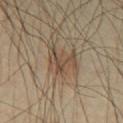Automated tile analysis of the lesion measured a lesion area of about 8.5 mm², an outline eccentricity of about 0.55 (0 = round, 1 = elongated), and a symmetry-axis asymmetry near 0.5. The analysis additionally found a lesion color around L≈48 a*≈14 b*≈28 in CIELAB and a lesion–skin lightness drop of about 8. The software also gave border irregularity of about 7 on a 0–10 scale and a within-lesion color-variation index near 4/10.
The lesion is located on the chest.
A male patient, aged around 40.
A 15 mm close-up tile from a total-body photography series done for melanoma screening.
About 4 mm across.
Captured under cross-polarized illumination.